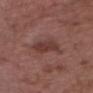  biopsy_status: not biopsied; imaged during a skin examination
  lesion_size:
    long_diameter_mm_approx: 4.0
  site: head or neck
  patient:
    sex: male
    age_approx: 50
  image:
    source: total-body photography crop
    field_of_view_mm: 15
  automated_metrics:
    cielab_L: 38
    cielab_a: 21
    cielab_b: 22
    vs_skin_darker_L: 8.0
    color_variation_0_10: 2.5
    peripheral_color_asymmetry: 1.0
    nevus_likeness_0_100: 15